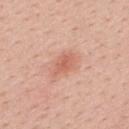Captured during whole-body skin photography for melanoma surveillance; the lesion was not biopsied.
Located on the upper back.
Captured under white-light illumination.
Measured at roughly 3.5 mm in maximum diameter.
A lesion tile, about 15 mm wide, cut from a 3D total-body photograph.
The subject is a female in their 40s.